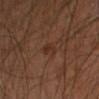Clinical impression:
Part of a total-body skin-imaging series; this lesion was reviewed on a skin check and was not flagged for biopsy.
Background:
Approximately 2.5 mm at its widest. Captured under cross-polarized illumination. A close-up tile cropped from a whole-body skin photograph, about 15 mm across. A male subject, aged approximately 45. An algorithmic analysis of the crop reported an area of roughly 2.5 mm² and an outline eccentricity of about 0.9 (0 = round, 1 = elongated). And it measured an automated nevus-likeness rating near 10 out of 100 and a lesion-detection confidence of about 100/100. From the left upper arm.